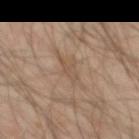biopsy status: imaged on a skin check; not biopsied | imaging modality: ~15 mm crop, total-body skin-cancer survey | site: the front of the torso | subject: male, in their mid- to late 60s | lesion diameter: about 2.5 mm.